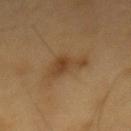Part of a total-body skin-imaging series; this lesion was reviewed on a skin check and was not flagged for biopsy.
A region of skin cropped from a whole-body photographic capture, roughly 15 mm wide.
On the mid back.
A male subject approximately 60 years of age.
Automated image analysis of the tile measured a footprint of about 9 mm², an outline eccentricity of about 0.8 (0 = round, 1 = elongated), and a symmetry-axis asymmetry near 0.45. It also reported a classifier nevus-likeness of about 30/100 and a lesion-detection confidence of about 100/100.
About 4.5 mm across.
Captured under cross-polarized illumination.